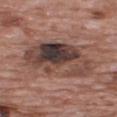| feature | finding |
|---|---|
| follow-up | total-body-photography surveillance lesion; no biopsy |
| site | the upper back |
| lesion size | ≈8 mm |
| automated metrics | a shape eccentricity near 0.8; an average lesion color of about L≈41 a*≈17 b*≈21 (CIELAB) and a lesion-to-skin contrast of about 10.5 (normalized; higher = more distinct); a classifier nevus-likeness of about 10/100 and a lesion-detection confidence of about 100/100 |
| image source | 15 mm crop, total-body photography |
| tile lighting | white-light |
| subject | male, about 70 years old |